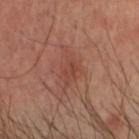follow-up = no biopsy performed (imaged during a skin exam)